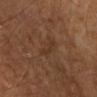Clinical impression:
Recorded during total-body skin imaging; not selected for excision or biopsy.
Image and clinical context:
Measured at roughly 3.5 mm in maximum diameter. A male subject, aged around 70. Imaged with cross-polarized lighting. From the left upper arm. A 15 mm close-up tile from a total-body photography series done for melanoma screening.